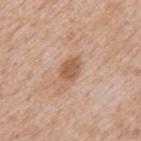| feature | finding |
|---|---|
| follow-up | no biopsy performed (imaged during a skin exam) |
| imaging modality | total-body-photography crop, ~15 mm field of view |
| patient | male, aged around 65 |
| size | about 4 mm |
| illumination | white-light illumination |
| location | the mid back |